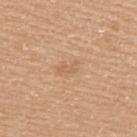Impression: Captured during whole-body skin photography for melanoma surveillance; the lesion was not biopsied. Clinical summary: An algorithmic analysis of the crop reported an average lesion color of about L≈61 a*≈20 b*≈36 (CIELAB), roughly 7 lightness units darker than nearby skin, and a normalized border contrast of about 4.5. It also reported a border-irregularity rating of about 5/10, a color-variation rating of about 0/10, and peripheral color asymmetry of about 0. The software also gave an automated nevus-likeness rating near 0 out of 100 and a lesion-detection confidence of about 100/100. The subject is a male about 40 years old. The lesion is located on the upper back. Cropped from a total-body skin-imaging series; the visible field is about 15 mm. Imaged with white-light lighting.